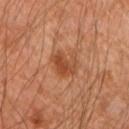This lesion was catalogued during total-body skin photography and was not selected for biopsy.
Imaged with cross-polarized lighting.
The lesion is on the left forearm.
A roughly 15 mm field-of-view crop from a total-body skin photograph.
A male patient, in their mid-40s.
The lesion's longest dimension is about 3.5 mm.